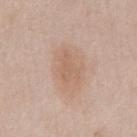Part of a total-body skin-imaging series; this lesion was reviewed on a skin check and was not flagged for biopsy.
Located on the chest.
The lesion-visualizer software estimated an average lesion color of about L≈61 a*≈18 b*≈30 (CIELAB), roughly 7 lightness units darker than nearby skin, and a lesion-to-skin contrast of about 5 (normalized; higher = more distinct). The analysis additionally found a border-irregularity index near 4.5/10, internal color variation of about 1.5 on a 0–10 scale, and radial color variation of about 0.5.
About 4 mm across.
A male subject in their 60s.
A region of skin cropped from a whole-body photographic capture, roughly 15 mm wide.